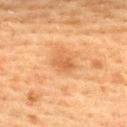Notes:
• acquisition — total-body-photography crop, ~15 mm field of view
• site — the upper back
• patient — female, aged 53 to 57